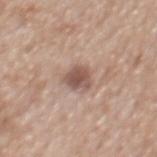Imaged during a routine full-body skin examination; the lesion was not biopsied and no histopathology is available.